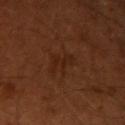Acquisition and patient details:
A male subject aged 48 to 52. This image is a 15 mm lesion crop taken from a total-body photograph. Imaged with cross-polarized lighting. From the arm. The recorded lesion diameter is about 3.5 mm.